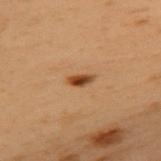No biopsy was performed on this lesion — it was imaged during a full skin examination and was not determined to be concerning. On the back. A female subject approximately 60 years of age. About 3 mm across. Cropped from a total-body skin-imaging series; the visible field is about 15 mm. Imaged with cross-polarized lighting.